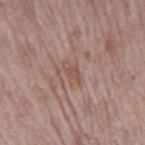biopsy status: total-body-photography surveillance lesion; no biopsy
patient: male, about 70 years old
location: the back
lesion size: ≈2.5 mm
image: ~15 mm tile from a whole-body skin photo
lighting: white-light